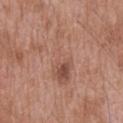Clinical impression: This lesion was catalogued during total-body skin photography and was not selected for biopsy. Context: A male patient, in their 50s. The tile uses white-light illumination. A 15 mm close-up extracted from a 3D total-body photography capture. The lesion's longest dimension is about 7.5 mm. The lesion is on the mid back.